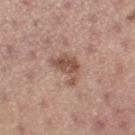Impression: This lesion was catalogued during total-body skin photography and was not selected for biopsy. Background: Imaged with white-light lighting. A female patient aged approximately 25. A 15 mm close-up tile from a total-body photography series done for melanoma screening. The lesion is located on the left thigh. Measured at roughly 4 mm in maximum diameter.